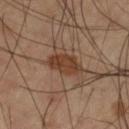Part of a total-body skin-imaging series; this lesion was reviewed on a skin check and was not flagged for biopsy. The lesion is located on the leg. A lesion tile, about 15 mm wide, cut from a 3D total-body photograph. The patient is a male aged 58–62.